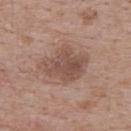  biopsy_status: not biopsied; imaged during a skin examination
  lighting: white-light
  patient:
    sex: male
    age_approx: 70
  image:
    source: total-body photography crop
    field_of_view_mm: 15
  automated_metrics:
    nevus_likeness_0_100: 25
  site: upper back
  lesion_size:
    long_diameter_mm_approx: 5.5